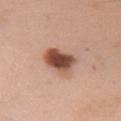Context:
From the right upper arm. A female patient aged 48–52. Cropped from a total-body skin-imaging series; the visible field is about 15 mm. About 4 mm across. The lesion-visualizer software estimated an area of roughly 10 mm² and a shape eccentricity near 0.7.